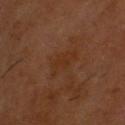<tbp_lesion>
  <biopsy_status>not biopsied; imaged during a skin examination</biopsy_status>
  <automated_metrics>
    <area_mm2_approx>5.0</area_mm2_approx>
    <eccentricity>0.85</eccentricity>
    <shape_asymmetry>0.35</shape_asymmetry>
    <cielab_L>29</cielab_L>
    <cielab_a>19</cielab_a>
    <cielab_b>29</cielab_b>
    <vs_skin_darker_L>4.0</vs_skin_darker_L>
    <vs_skin_contrast_norm>5.5</vs_skin_contrast_norm>
    <border_irregularity_0_10>5.0</border_irregularity_0_10>
    <color_variation_0_10>1.0</color_variation_0_10>
    <peripheral_color_asymmetry>0.5</peripheral_color_asymmetry>
    <nevus_likeness_0_100>0</nevus_likeness_0_100>
  </automated_metrics>
  <patient>
    <sex>male</sex>
    <age_approx>60</age_approx>
  </patient>
  <image>
    <source>total-body photography crop</source>
    <field_of_view_mm>15</field_of_view_mm>
  </image>
  <site>head or neck</site>
  <lighting>cross-polarized</lighting>
</tbp_lesion>